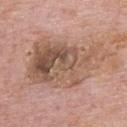A lesion tile, about 15 mm wide, cut from a 3D total-body photograph.
This is a white-light tile.
The lesion is on the back.
A male patient, approximately 75 years of age.
Automated image analysis of the tile measured a lesion area of about 41 mm² and an eccentricity of roughly 0.8. It also reported a border-irregularity rating of about 5/10 and peripheral color asymmetry of about 3.5.